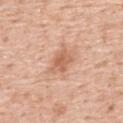notes: catalogued during a skin exam; not biopsied
imaging modality: ~15 mm tile from a whole-body skin photo
location: the upper back
patient: male, in their 60s